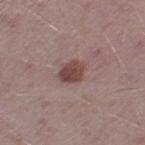workup: total-body-photography surveillance lesion; no biopsy | imaging modality: total-body-photography crop, ~15 mm field of view | site: the left thigh | lesion diameter: ~3 mm (longest diameter) | tile lighting: white-light illumination | patient: male, in their 40s.